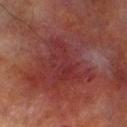Acquisition and patient details:
Longest diameter approximately 5 mm. The tile uses cross-polarized illumination. A male subject approximately 70 years of age. From the leg. A lesion tile, about 15 mm wide, cut from a 3D total-body photograph.